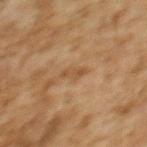follow-up: catalogued during a skin exam; not biopsied
image: 15 mm crop, total-body photography
diameter: ≈3 mm
patient: female, roughly 60 years of age
anatomic site: the upper back